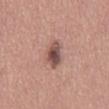This lesion was catalogued during total-body skin photography and was not selected for biopsy. On the mid back. A 15 mm close-up tile from a total-body photography series done for melanoma screening. Longest diameter approximately 4.5 mm. An algorithmic analysis of the crop reported a lesion color around L≈50 a*≈20 b*≈22 in CIELAB, a lesion–skin lightness drop of about 14, and a normalized lesion–skin contrast near 10. The analysis additionally found an automated nevus-likeness rating near 95 out of 100 and a detector confidence of about 100 out of 100 that the crop contains a lesion. Imaged with white-light lighting. The patient is a female aged around 50.